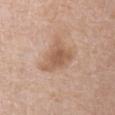patient=female, roughly 75 years of age; image source=~15 mm tile from a whole-body skin photo; location=the front of the torso; lesion diameter=~4.5 mm (longest diameter); tile lighting=white-light illumination.